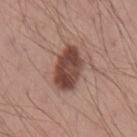This lesion was catalogued during total-body skin photography and was not selected for biopsy.
A 15 mm crop from a total-body photograph taken for skin-cancer surveillance.
The lesion is located on the left upper arm.
A male patient in their mid-30s.